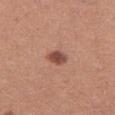Notes:
– notes — no biopsy performed (imaged during a skin exam)
– location — the leg
– image — ~15 mm tile from a whole-body skin photo
– subject — female, aged 38–42
– illumination — white-light illumination
– lesion diameter — ~2.5 mm (longest diameter)